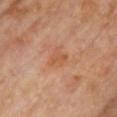Captured during whole-body skin photography for melanoma surveillance; the lesion was not biopsied. The lesion-visualizer software estimated a mean CIELAB color near L≈53 a*≈24 b*≈35, roughly 6 lightness units darker than nearby skin, and a normalized border contrast of about 5.5. It also reported a border-irregularity rating of about 4.5/10, internal color variation of about 1.5 on a 0–10 scale, and peripheral color asymmetry of about 0.5. The analysis additionally found a nevus-likeness score of about 0/100 and a detector confidence of about 100 out of 100 that the crop contains a lesion. The lesion is located on the chest. The tile uses cross-polarized illumination. A 15 mm crop from a total-body photograph taken for skin-cancer surveillance. The recorded lesion diameter is about 2.5 mm. A female subject approximately 65 years of age.